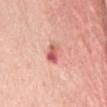Located on the chest.
A female subject aged around 75.
Cropped from a total-body skin-imaging series; the visible field is about 15 mm.
The tile uses white-light illumination.
An algorithmic analysis of the crop reported an automated nevus-likeness rating near 0 out of 100 and a lesion-detection confidence of about 100/100.
The recorded lesion diameter is about 3 mm.